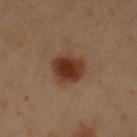Impression:
This lesion was catalogued during total-body skin photography and was not selected for biopsy.
Clinical summary:
About 4.5 mm across. This is a cross-polarized tile. Cropped from a whole-body photographic skin survey; the tile spans about 15 mm. A male patient, aged 48–52. From the chest.